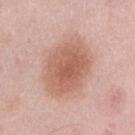Notes:
- follow-up — catalogued during a skin exam; not biopsied
- patient — female, aged 48 to 52
- imaging modality — total-body-photography crop, ~15 mm field of view
- TBP lesion metrics — a lesion area of about 28 mm² and two-axis asymmetry of about 0.1; a mean CIELAB color near L≈60 a*≈22 b*≈28, roughly 11 lightness units darker than nearby skin, and a normalized lesion–skin contrast near 7.5; an automated nevus-likeness rating near 95 out of 100 and a lesion-detection confidence of about 100/100
- diameter — ~6.5 mm (longest diameter)
- site — the lower back